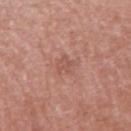This lesion was catalogued during total-body skin photography and was not selected for biopsy. Measured at roughly 3 mm in maximum diameter. A male subject, aged approximately 70. Located on the right upper arm. A 15 mm crop from a total-body photograph taken for skin-cancer surveillance. The lesion-visualizer software estimated an eccentricity of roughly 0.7. And it measured a lesion color around L≈54 a*≈24 b*≈27 in CIELAB, a lesion–skin lightness drop of about 6, and a lesion-to-skin contrast of about 4.5 (normalized; higher = more distinct). The software also gave a detector confidence of about 100 out of 100 that the crop contains a lesion. The tile uses white-light illumination.